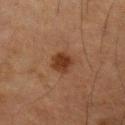{"biopsy_status": "not biopsied; imaged during a skin examination", "image": {"source": "total-body photography crop", "field_of_view_mm": 15}, "automated_metrics": {"area_mm2_approx": 5.5, "eccentricity": 0.7, "shape_asymmetry": 0.2, "border_irregularity_0_10": 1.5, "nevus_likeness_0_100": 95, "lesion_detection_confidence_0_100": 100}, "patient": {"sex": "male", "age_approx": 65}, "site": "left forearm"}